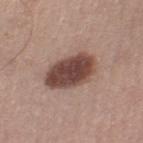Q: Was this lesion biopsied?
A: no biopsy performed (imaged during a skin exam)
Q: How was this image acquired?
A: ~15 mm crop, total-body skin-cancer survey
Q: Who is the patient?
A: male, in their 50s
Q: Automated lesion metrics?
A: a lesion area of about 17 mm², an outline eccentricity of about 0.8 (0 = round, 1 = elongated), and a symmetry-axis asymmetry near 0.15; a lesion color around L≈45 a*≈19 b*≈23 in CIELAB, roughly 17 lightness units darker than nearby skin, and a normalized border contrast of about 12; a border-irregularity index near 1.5/10, internal color variation of about 4.5 on a 0–10 scale, and radial color variation of about 1.5; a classifier nevus-likeness of about 85/100 and lesion-presence confidence of about 100/100
Q: What is the lesion's diameter?
A: about 6 mm
Q: Where on the body is the lesion?
A: the right thigh